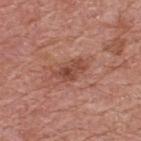biopsy status: total-body-photography surveillance lesion; no biopsy | automated metrics: a lesion color around L≈47 a*≈25 b*≈28 in CIELAB and roughly 10 lightness units darker than nearby skin; a border-irregularity rating of about 4/10 and radial color variation of about 1.5 | acquisition: 15 mm crop, total-body photography | anatomic site: the upper back | lesion diameter: ≈3.5 mm | patient: male, approximately 70 years of age | tile lighting: white-light illumination.